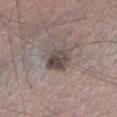{"biopsy_status": "not biopsied; imaged during a skin examination", "automated_metrics": {"area_mm2_approx": 9.0, "eccentricity": 0.5, "shape_asymmetry": 0.15}, "image": {"source": "total-body photography crop", "field_of_view_mm": 15}, "site": "left lower leg", "lighting": "white-light", "patient": {"sex": "male", "age_approx": 45}, "lesion_size": {"long_diameter_mm_approx": 3.5}}